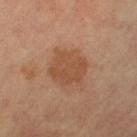biopsy status: imaged on a skin check; not biopsied
imaging modality: ~15 mm crop, total-body skin-cancer survey
lighting: cross-polarized illumination
automated metrics: two-axis asymmetry of about 0.2; a nevus-likeness score of about 30/100 and a lesion-detection confidence of about 100/100
anatomic site: the left leg
patient: female, approximately 60 years of age
lesion size: ~4 mm (longest diameter)